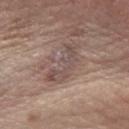Clinical impression:
Part of a total-body skin-imaging series; this lesion was reviewed on a skin check and was not flagged for biopsy.
Clinical summary:
The lesion is located on the left forearm. The recorded lesion diameter is about 5 mm. The tile uses white-light illumination. A male subject, aged 68–72. A lesion tile, about 15 mm wide, cut from a 3D total-body photograph.